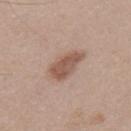Q: Is there a histopathology result?
A: total-body-photography surveillance lesion; no biopsy
Q: Who is the patient?
A: male, about 40 years old
Q: How was the tile lit?
A: white-light illumination
Q: What kind of image is this?
A: total-body-photography crop, ~15 mm field of view
Q: Where on the body is the lesion?
A: the upper back
Q: What did automated image analysis measure?
A: a footprint of about 9 mm² and an outline eccentricity of about 0.8 (0 = round, 1 = elongated); about 11 CIELAB-L* units darker than the surrounding skin and a normalized border contrast of about 8; lesion-presence confidence of about 100/100
Q: How large is the lesion?
A: ≈4.5 mm